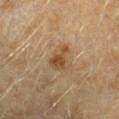<tbp_lesion>
  <biopsy_status>not biopsied; imaged during a skin examination</biopsy_status>
  <site>left forearm</site>
  <patient>
    <sex>female</sex>
    <age_approx>60</age_approx>
  </patient>
  <image>
    <source>total-body photography crop</source>
    <field_of_view_mm>15</field_of_view_mm>
  </image>
</tbp_lesion>